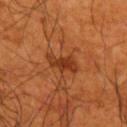Imaged during a routine full-body skin examination; the lesion was not biopsied and no histopathology is available.